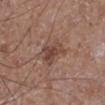Recorded during total-body skin imaging; not selected for excision or biopsy. The lesion's longest dimension is about 3.5 mm. A 15 mm close-up extracted from a 3D total-body photography capture. The lesion is located on the leg. The subject is a male in their mid- to late 60s. Automated tile analysis of the lesion measured a footprint of about 7 mm², an eccentricity of roughly 0.65, and a shape-asymmetry score of about 0.45 (0 = symmetric). It also reported a border-irregularity rating of about 5/10, a within-lesion color-variation index near 4/10, and a peripheral color-asymmetry measure near 1.5. The analysis additionally found a classifier nevus-likeness of about 5/100 and a detector confidence of about 100 out of 100 that the crop contains a lesion.